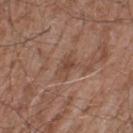* notes · imaged on a skin check; not biopsied
* tile lighting · white-light illumination
* imaging modality · ~15 mm crop, total-body skin-cancer survey
* image-analysis metrics · border irregularity of about 3.5 on a 0–10 scale, a color-variation rating of about 2.5/10, and radial color variation of about 0.5; an automated nevus-likeness rating near 0 out of 100 and lesion-presence confidence of about 100/100
* location · the leg
* patient · male, aged 58 to 62